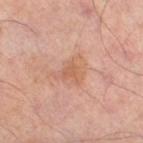<record>
<biopsy_status>not biopsied; imaged during a skin examination</biopsy_status>
<site>leg</site>
<patient>
  <sex>male</sex>
  <age_approx>65</age_approx>
</patient>
<lighting>cross-polarized</lighting>
<image>
  <source>total-body photography crop</source>
  <field_of_view_mm>15</field_of_view_mm>
</image>
</record>